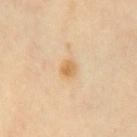The lesion was tiled from a total-body skin photograph and was not biopsied. A female patient, in their mid- to late 50s. Automated image analysis of the tile measured a footprint of about 4 mm². The software also gave internal color variation of about 3.5 on a 0–10 scale and peripheral color asymmetry of about 1. Imaged with cross-polarized lighting. Measured at roughly 2.5 mm in maximum diameter. From the chest. Cropped from a whole-body photographic skin survey; the tile spans about 15 mm.